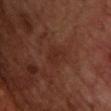This lesion was catalogued during total-body skin photography and was not selected for biopsy. Longest diameter approximately 3 mm. The lesion is on the upper back. A male patient aged 68–72. Cropped from a total-body skin-imaging series; the visible field is about 15 mm.